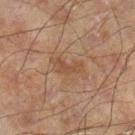A lesion tile, about 15 mm wide, cut from a 3D total-body photograph. The patient is a male aged around 60. From the left leg.